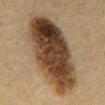Assessment:
The lesion was photographed on a routine skin check and not biopsied; there is no pathology result.
Acquisition and patient details:
Imaged with cross-polarized lighting. From the mid back. Cropped from a total-body skin-imaging series; the visible field is about 15 mm. Automated image analysis of the tile measured a mean CIELAB color near L≈34 a*≈13 b*≈26, a lesion–skin lightness drop of about 17, and a normalized lesion–skin contrast near 14. It also reported border irregularity of about 2 on a 0–10 scale and a color-variation rating of about 9.5/10. The software also gave a classifier nevus-likeness of about 100/100 and a detector confidence of about 100 out of 100 that the crop contains a lesion. The subject is a male roughly 60 years of age.